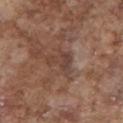The lesion was tiled from a total-body skin photograph and was not biopsied. A male patient, approximately 75 years of age. A 15 mm close-up tile from a total-body photography series done for melanoma screening. This is a white-light tile. The lesion is located on the chest. Automated tile analysis of the lesion measured a lesion area of about 5.5 mm², an outline eccentricity of about 0.8 (0 = round, 1 = elongated), and a symmetry-axis asymmetry near 0.6. It also reported a mean CIELAB color near L≈41 a*≈18 b*≈25, roughly 7 lightness units darker than nearby skin, and a normalized lesion–skin contrast near 6. The analysis additionally found a classifier nevus-likeness of about 0/100 and lesion-presence confidence of about 75/100. Measured at roughly 4 mm in maximum diameter.